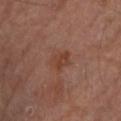workup=no biopsy performed (imaged during a skin exam)
image=~15 mm tile from a whole-body skin photo
subject=male, aged around 70
tile lighting=cross-polarized illumination
lesion size=~3 mm (longest diameter)
image-analysis metrics=a footprint of about 4.5 mm² and a symmetry-axis asymmetry near 0.3; about 6 CIELAB-L* units darker than the surrounding skin; a color-variation rating of about 2/10 and peripheral color asymmetry of about 1; a classifier nevus-likeness of about 0/100 and lesion-presence confidence of about 100/100
site=the left lower leg